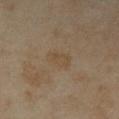No biopsy was performed on this lesion — it was imaged during a full skin examination and was not determined to be concerning. An algorithmic analysis of the crop reported a footprint of about 5.5 mm² and a shape eccentricity near 0.75. And it measured a color-variation rating of about 1.5/10. Captured under cross-polarized illumination. A female subject aged approximately 35. The recorded lesion diameter is about 3 mm. Cropped from a total-body skin-imaging series; the visible field is about 15 mm. From the right forearm.